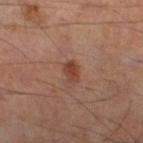Impression: This lesion was catalogued during total-body skin photography and was not selected for biopsy. Image and clinical context: A lesion tile, about 15 mm wide, cut from a 3D total-body photograph. This is a cross-polarized tile. The lesion is located on the left lower leg. A male subject, approximately 55 years of age. Measured at roughly 2.5 mm in maximum diameter.